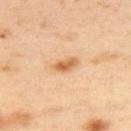This lesion was catalogued during total-body skin photography and was not selected for biopsy. The recorded lesion diameter is about 3.5 mm. The lesion is on the upper back. A 15 mm close-up extracted from a 3D total-body photography capture. Captured under cross-polarized illumination. A female patient about 40 years old.